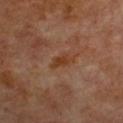Assessment:
Part of a total-body skin-imaging series; this lesion was reviewed on a skin check and was not flagged for biopsy.
Image and clinical context:
Located on the chest. A female patient about 60 years old. A region of skin cropped from a whole-body photographic capture, roughly 15 mm wide. This is a cross-polarized tile. Longest diameter approximately 3 mm. Automated tile analysis of the lesion measured an area of roughly 3.5 mm², an eccentricity of roughly 0.9, and a shape-asymmetry score of about 0.35 (0 = symmetric). It also reported border irregularity of about 3.5 on a 0–10 scale, a color-variation rating of about 2/10, and peripheral color asymmetry of about 0.5. And it measured a nevus-likeness score of about 0/100 and lesion-presence confidence of about 100/100.